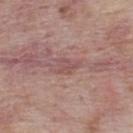follow-up: total-body-photography surveillance lesion; no biopsy